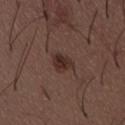Captured during whole-body skin photography for melanoma surveillance; the lesion was not biopsied. Cropped from a total-body skin-imaging series; the visible field is about 15 mm. A male subject aged 48–52. The lesion is located on the abdomen. An algorithmic analysis of the crop reported a classifier nevus-likeness of about 95/100.